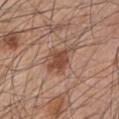The lesion was tiled from a total-body skin photograph and was not biopsied.
The recorded lesion diameter is about 5 mm.
The lesion is on the arm.
An algorithmic analysis of the crop reported a shape eccentricity near 0.75 and a symmetry-axis asymmetry near 0.35. The analysis additionally found a lesion color around L≈49 a*≈20 b*≈28 in CIELAB, a lesion–skin lightness drop of about 10, and a lesion-to-skin contrast of about 7 (normalized; higher = more distinct).
A male subject, aged approximately 45.
Cropped from a whole-body photographic skin survey; the tile spans about 15 mm.
Captured under white-light illumination.